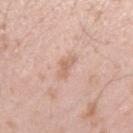The lesion was photographed on a routine skin check and not biopsied; there is no pathology result.
A 15 mm close-up tile from a total-body photography series done for melanoma screening.
This is a white-light tile.
Longest diameter approximately 2.5 mm.
The lesion is located on the left forearm.
Automated tile analysis of the lesion measured a footprint of about 3.5 mm², an outline eccentricity of about 0.8 (0 = round, 1 = elongated), and a shape-asymmetry score of about 0.4 (0 = symmetric). The software also gave a lesion–skin lightness drop of about 8 and a normalized lesion–skin contrast near 5.5.
The subject is a male aged around 25.